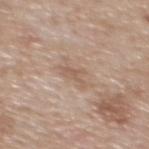The lesion was tiled from a total-body skin photograph and was not biopsied.
The patient is a female aged approximately 40.
A 15 mm close-up extracted from a 3D total-body photography capture.
Located on the upper back.